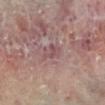Notes:
- notes — imaged on a skin check; not biopsied
- body site — the left lower leg
- imaging modality — ~15 mm crop, total-body skin-cancer survey
- subject — female, aged around 55
- TBP lesion metrics — two-axis asymmetry of about 0.35; an average lesion color of about L≈51 a*≈21 b*≈18 (CIELAB), a lesion–skin lightness drop of about 7, and a normalized border contrast of about 5.5; a border-irregularity rating of about 4/10, a color-variation rating of about 1.5/10, and radial color variation of about 0.5; a nevus-likeness score of about 0/100 and a detector confidence of about 80 out of 100 that the crop contains a lesion
- size — about 3 mm